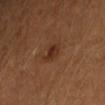follow-up = no biopsy performed (imaged during a skin exam)
image = 15 mm crop, total-body photography
image-analysis metrics = a border-irregularity rating of about 3/10, a color-variation rating of about 3.5/10, and peripheral color asymmetry of about 1
size = ~2.5 mm (longest diameter)
patient = male, roughly 40 years of age
anatomic site = the left forearm